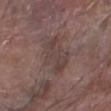Part of a total-body skin-imaging series; this lesion was reviewed on a skin check and was not flagged for biopsy. Located on the left lower leg. Approximately 4.5 mm at its widest. A male patient, approximately 80 years of age. Cropped from a total-body skin-imaging series; the visible field is about 15 mm.